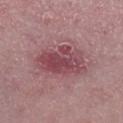The lesion's longest dimension is about 6 mm. A female subject, roughly 45 years of age. Located on the left lower leg. This image is a 15 mm lesion crop taken from a total-body photograph. The tile uses white-light illumination.